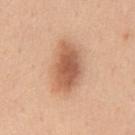* workup — total-body-photography surveillance lesion; no biopsy
* TBP lesion metrics — a mean CIELAB color near L≈59 a*≈23 b*≈33, a lesion–skin lightness drop of about 14, and a lesion-to-skin contrast of about 8.5 (normalized; higher = more distinct); a classifier nevus-likeness of about 100/100 and a lesion-detection confidence of about 100/100
* acquisition — ~15 mm tile from a whole-body skin photo
* lighting — white-light illumination
* body site — the abdomen
* subject — male, aged approximately 50